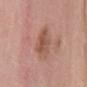imaging modality = total-body-photography crop, ~15 mm field of view; patient = female, approximately 50 years of age; site = the mid back.